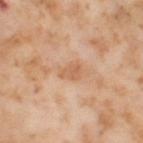Assessment:
Part of a total-body skin-imaging series; this lesion was reviewed on a skin check and was not flagged for biopsy.
Acquisition and patient details:
An algorithmic analysis of the crop reported a mean CIELAB color near L≈62 a*≈22 b*≈35, about 8 CIELAB-L* units darker than the surrounding skin, and a normalized lesion–skin contrast near 5.5. It also reported a border-irregularity rating of about 2.5/10. And it measured a classifier nevus-likeness of about 0/100 and lesion-presence confidence of about 100/100. Measured at roughly 2.5 mm in maximum diameter. The subject is a female in their mid-50s. The lesion is on the left thigh. A 15 mm crop from a total-body photograph taken for skin-cancer surveillance. The tile uses cross-polarized illumination.